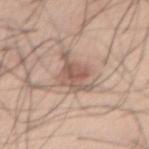<case>
<biopsy_status>not biopsied; imaged during a skin examination</biopsy_status>
<lighting>white-light</lighting>
<site>front of the torso</site>
<image>
  <source>total-body photography crop</source>
  <field_of_view_mm>15</field_of_view_mm>
</image>
<patient>
  <sex>male</sex>
  <age_approx>55</age_approx>
</patient>
</case>